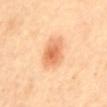Impression: Captured during whole-body skin photography for melanoma surveillance; the lesion was not biopsied. Acquisition and patient details: Located on the mid back. A female patient, aged approximately 60. Cropped from a whole-body photographic skin survey; the tile spans about 15 mm. The recorded lesion diameter is about 4 mm. The total-body-photography lesion software estimated an area of roughly 10 mm², a shape eccentricity near 0.7, and two-axis asymmetry of about 0.25. It also reported a border-irregularity rating of about 2/10 and peripheral color asymmetry of about 1.5. The tile uses cross-polarized illumination.